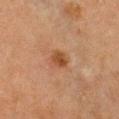follow-up = catalogued during a skin exam; not biopsied
anatomic site = the front of the torso
automated lesion analysis = a mean CIELAB color near L≈39 a*≈20 b*≈30, roughly 8 lightness units darker than nearby skin, and a normalized lesion–skin contrast near 7.5
subject = female, about 60 years old
lighting = cross-polarized
image source = ~15 mm crop, total-body skin-cancer survey
lesion diameter = about 2.5 mm